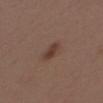The lesion is located on the back.
Longest diameter approximately 2.5 mm.
Cropped from a whole-body photographic skin survey; the tile spans about 15 mm.
Imaged with white-light lighting.
A male patient, aged 38–42.